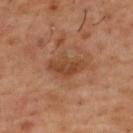subject = male, aged 53 to 57
TBP lesion metrics = border irregularity of about 3 on a 0–10 scale, a color-variation rating of about 2.5/10, and a peripheral color-asymmetry measure near 0.5; an automated nevus-likeness rating near 0 out of 100 and lesion-presence confidence of about 100/100
lesion size = about 4.5 mm
location = the upper back
imaging modality = ~15 mm tile from a whole-body skin photo